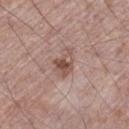| key | value |
|---|---|
| biopsy status | catalogued during a skin exam; not biopsied |
| site | the left thigh |
| image source | ~15 mm crop, total-body skin-cancer survey |
| patient | male, aged 68–72 |
| lesion diameter | about 3.5 mm |
| illumination | white-light |